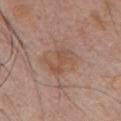Findings:
- workup · imaged on a skin check; not biopsied